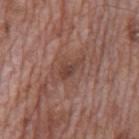Clinical impression:
Recorded during total-body skin imaging; not selected for excision or biopsy.
Background:
Longest diameter approximately 3 mm. The lesion is on the mid back. A region of skin cropped from a whole-body photographic capture, roughly 15 mm wide. A male patient roughly 70 years of age. The tile uses white-light illumination.